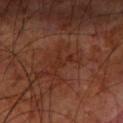follow-up = total-body-photography surveillance lesion; no biopsy.